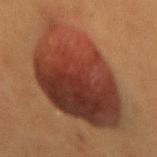Assessment:
No biopsy was performed on this lesion — it was imaged during a full skin examination and was not determined to be concerning.
Clinical summary:
From the back. Captured under cross-polarized illumination. A female patient aged 48–52. Longest diameter approximately 13 mm. Cropped from a total-body skin-imaging series; the visible field is about 15 mm.